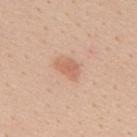{
  "biopsy_status": "not biopsied; imaged during a skin examination",
  "image": {
    "source": "total-body photography crop",
    "field_of_view_mm": 15
  },
  "site": "upper back",
  "automated_metrics": {
    "vs_skin_darker_L": 9.0,
    "nevus_likeness_0_100": 60,
    "lesion_detection_confidence_0_100": 100
  },
  "lesion_size": {
    "long_diameter_mm_approx": 3.0
  },
  "patient": {
    "sex": "female",
    "age_approx": 45
  }
}